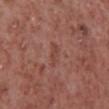follow-up = total-body-photography surveillance lesion; no biopsy | anatomic site = the right lower leg | image = ~15 mm crop, total-body skin-cancer survey | diameter = ≈3 mm | tile lighting = white-light | subject = male, aged around 55 | automated metrics = an eccentricity of roughly 0.9 and a symmetry-axis asymmetry near 0.35; a mean CIELAB color near L≈44 a*≈24 b*≈25, a lesion–skin lightness drop of about 6, and a normalized lesion–skin contrast near 5; a lesion-detection confidence of about 100/100.